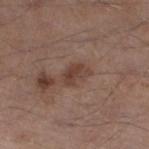workup: imaged on a skin check; not biopsied
patient: male, aged 53–57
automated lesion analysis: a lesion area of about 4.5 mm², an outline eccentricity of about 0.8 (0 = round, 1 = elongated), and a symmetry-axis asymmetry near 0.3; an average lesion color of about L≈41 a*≈18 b*≈24 (CIELAB), roughly 9 lightness units darker than nearby skin, and a normalized border contrast of about 7.5; a color-variation rating of about 2.5/10 and radial color variation of about 1; an automated nevus-likeness rating near 10 out of 100 and lesion-presence confidence of about 100/100
acquisition: ~15 mm crop, total-body skin-cancer survey
size: ≈3 mm
location: the left lower leg
tile lighting: white-light illumination